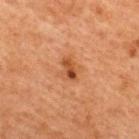workup: imaged on a skin check; not biopsied
anatomic site: the upper back
acquisition: ~15 mm crop, total-body skin-cancer survey
lesion size: ~2.5 mm (longest diameter)
lighting: cross-polarized
TBP lesion metrics: a mean CIELAB color near L≈44 a*≈24 b*≈35, about 10 CIELAB-L* units darker than the surrounding skin, and a lesion-to-skin contrast of about 8 (normalized; higher = more distinct); border irregularity of about 3 on a 0–10 scale, a within-lesion color-variation index near 7/10, and peripheral color asymmetry of about 2.5
patient: female, in their 40s